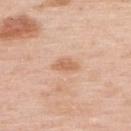biopsy_status: not biopsied; imaged during a skin examination
image:
  source: total-body photography crop
  field_of_view_mm: 15
patient:
  sex: female
  age_approx: 50
site: upper back